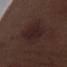notes: imaged on a skin check; not biopsied | body site: the left upper arm | subject: male, aged around 70 | automated metrics: a footprint of about 11 mm²; a lesion color around L≈21 a*≈17 b*≈15 in CIELAB, about 6 CIELAB-L* units darker than the surrounding skin, and a normalized lesion–skin contrast near 8; a classifier nevus-likeness of about 55/100 and a detector confidence of about 100 out of 100 that the crop contains a lesion | lighting: white-light illumination | imaging modality: ~15 mm tile from a whole-body skin photo.